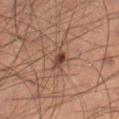biopsy_status: not biopsied; imaged during a skin examination
site: right thigh
image:
  source: total-body photography crop
  field_of_view_mm: 15
lesion_size:
  long_diameter_mm_approx: 2.5
automated_metrics:
  eccentricity: 0.75
  shape_asymmetry: 0.25
  cielab_L: 39
  cielab_a: 20
  cielab_b: 25
  vs_skin_contrast_norm: 9.0
  border_irregularity_0_10: 2.0
  color_variation_0_10: 4.5
  peripheral_color_asymmetry: 1.5
  nevus_likeness_0_100: 90
  lesion_detection_confidence_0_100: 100
lighting: cross-polarized
patient:
  sex: male
  age_approx: 60